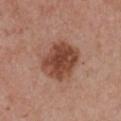biopsy status — total-body-photography surveillance lesion; no biopsy
anatomic site — the chest
patient — female, in their mid-50s
lesion diameter — about 5.5 mm
lighting — white-light illumination
image-analysis metrics — a mean CIELAB color near L≈45 a*≈23 b*≈29 and a lesion–skin lightness drop of about 13; border irregularity of about 2 on a 0–10 scale, a within-lesion color-variation index near 4.5/10, and peripheral color asymmetry of about 1.5; a classifier nevus-likeness of about 50/100 and a lesion-detection confidence of about 100/100
image — 15 mm crop, total-body photography